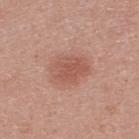Imaged during a routine full-body skin examination; the lesion was not biopsied and no histopathology is available. This image is a 15 mm lesion crop taken from a total-body photograph. Longest diameter approximately 4.5 mm. The subject is a male approximately 25 years of age. The tile uses white-light illumination. From the upper back. The lesion-visualizer software estimated an area of roughly 12 mm². It also reported an automated nevus-likeness rating near 70 out of 100 and a lesion-detection confidence of about 100/100.